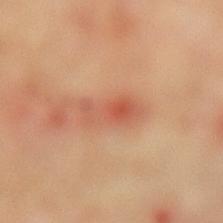Q: Is there a histopathology result?
A: total-body-photography surveillance lesion; no biopsy
Q: Where on the body is the lesion?
A: the leg
Q: Patient demographics?
A: male, aged approximately 65
Q: How was this image acquired?
A: ~15 mm tile from a whole-body skin photo
Q: Automated lesion metrics?
A: a shape eccentricity near 0.9 and two-axis asymmetry of about 0.3; a lesion-to-skin contrast of about 6 (normalized; higher = more distinct); a border-irregularity index near 4/10 and a color-variation rating of about 7/10; an automated nevus-likeness rating near 0 out of 100